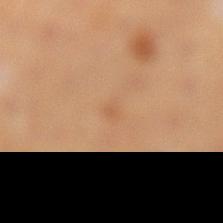Impression: Captured during whole-body skin photography for melanoma surveillance; the lesion was not biopsied. Clinical summary: The lesion is located on the leg. The lesion's longest dimension is about 1 mm. This image is a 15 mm lesion crop taken from a total-body photograph. An algorithmic analysis of the crop reported a lesion color around L≈56 a*≈21 b*≈36 in CIELAB. And it measured a border-irregularity index near 2.5/10, internal color variation of about 0 on a 0–10 scale, and a peripheral color-asymmetry measure near 0. And it measured a nevus-likeness score of about 0/100 and a lesion-detection confidence of about 100/100. A female subject, aged approximately 50.